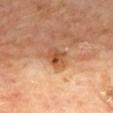This lesion was catalogued during total-body skin photography and was not selected for biopsy. The total-body-photography lesion software estimated border irregularity of about 2 on a 0–10 scale, internal color variation of about 5 on a 0–10 scale, and a peripheral color-asymmetry measure near 2. Longest diameter approximately 2.5 mm. The tile uses cross-polarized illumination. The subject is a male roughly 70 years of age. Located on the mid back. A roughly 15 mm field-of-view crop from a total-body skin photograph.